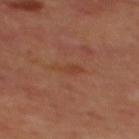A lesion tile, about 15 mm wide, cut from a 3D total-body photograph.
The lesion's longest dimension is about 3 mm.
The tile uses cross-polarized illumination.
The total-body-photography lesion software estimated an area of roughly 4 mm² and a shape-asymmetry score of about 0.3 (0 = symmetric). The analysis additionally found a lesion color around L≈39 a*≈22 b*≈30 in CIELAB and roughly 4 lightness units darker than nearby skin.
A male subject roughly 70 years of age.
Located on the mid back.